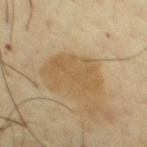Clinical impression:
Captured during whole-body skin photography for melanoma surveillance; the lesion was not biopsied.
Acquisition and patient details:
A region of skin cropped from a whole-body photographic capture, roughly 15 mm wide. A male patient, aged around 65. Imaged with cross-polarized lighting. An algorithmic analysis of the crop reported a lesion area of about 16 mm². The analysis additionally found a mean CIELAB color near L≈56 a*≈13 b*≈38, about 7 CIELAB-L* units darker than the surrounding skin, and a normalized lesion–skin contrast near 6. And it measured an automated nevus-likeness rating near 0 out of 100. Approximately 5.5 mm at its widest. The lesion is on the chest.